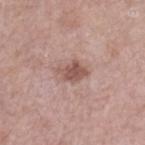{"biopsy_status": "not biopsied; imaged during a skin examination", "image": {"source": "total-body photography crop", "field_of_view_mm": 15}, "site": "right lower leg", "lighting": "white-light", "lesion_size": {"long_diameter_mm_approx": 3.0}, "patient": {"sex": "female", "age_approx": 65}}